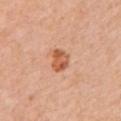Approximately 3 mm at its widest.
A female patient aged 53–57.
A 15 mm close-up tile from a total-body photography series done for melanoma screening.
An algorithmic analysis of the crop reported a within-lesion color-variation index near 4/10.
The lesion is on the left upper arm.